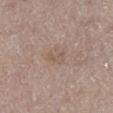No biopsy was performed on this lesion — it was imaged during a full skin examination and was not determined to be concerning. The lesion-visualizer software estimated a lesion area of about 4.5 mm², a shape eccentricity near 0.75, and two-axis asymmetry of about 0.3. The software also gave a lesion color around L≈55 a*≈15 b*≈25 in CIELAB and about 6 CIELAB-L* units darker than the surrounding skin. And it measured a border-irregularity index near 4/10, a within-lesion color-variation index near 2/10, and peripheral color asymmetry of about 1. The software also gave a nevus-likeness score of about 0/100 and a lesion-detection confidence of about 100/100. This is a white-light tile. This image is a 15 mm lesion crop taken from a total-body photograph. Located on the right thigh. Longest diameter approximately 3 mm. A male patient approximately 75 years of age.